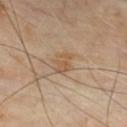notes = imaged on a skin check; not biopsied | patient = male, aged 63 to 67 | size = about 2.5 mm | anatomic site = the chest | TBP lesion metrics = a within-lesion color-variation index near 2.5/10 and a peripheral color-asymmetry measure near 0.5; a nevus-likeness score of about 0/100 and a lesion-detection confidence of about 100/100 | lighting = cross-polarized illumination | image = ~15 mm tile from a whole-body skin photo.